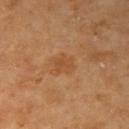Assessment: This lesion was catalogued during total-body skin photography and was not selected for biopsy. Context: On the right upper arm. The patient is a female roughly 70 years of age. Longest diameter approximately 3 mm. A region of skin cropped from a whole-body photographic capture, roughly 15 mm wide. Captured under cross-polarized illumination.